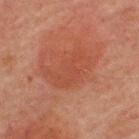The lesion was photographed on a routine skin check and not biopsied; there is no pathology result. Located on the back. The total-body-photography lesion software estimated an area of roughly 14 mm², an outline eccentricity of about 0.8 (0 = round, 1 = elongated), and a symmetry-axis asymmetry near 0.4. The software also gave border irregularity of about 5 on a 0–10 scale and a color-variation rating of about 2.5/10. This is a cross-polarized tile. A male patient aged 58–62. A region of skin cropped from a whole-body photographic capture, roughly 15 mm wide. Approximately 5.5 mm at its widest.